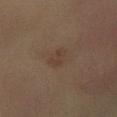Recorded during total-body skin imaging; not selected for excision or biopsy. Longest diameter approximately 2.5 mm. Located on the left leg. A roughly 15 mm field-of-view crop from a total-body skin photograph. The tile uses cross-polarized illumination. An algorithmic analysis of the crop reported a lesion area of about 3.5 mm², a shape eccentricity near 0.8, and a symmetry-axis asymmetry near 0.35. The patient is a female roughly 65 years of age.